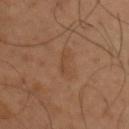Findings:
- illumination — cross-polarized illumination
- acquisition — 15 mm crop, total-body photography
- lesion diameter — about 2.5 mm
- anatomic site — the left upper arm
- patient — male, aged 48–52
- image-analysis metrics — a mean CIELAB color near L≈43 a*≈19 b*≈32 and about 5 CIELAB-L* units darker than the surrounding skin; an automated nevus-likeness rating near 0 out of 100